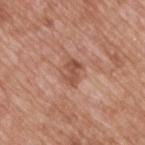Q: Was a biopsy performed?
A: no biopsy performed (imaged during a skin exam)
Q: How was this image acquired?
A: ~15 mm tile from a whole-body skin photo
Q: Illumination type?
A: white-light illumination
Q: How large is the lesion?
A: about 3 mm
Q: Lesion location?
A: the upper back
Q: What are the patient's age and sex?
A: male, about 50 years old